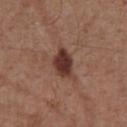Q: Was a biopsy performed?
A: imaged on a skin check; not biopsied
Q: What is the lesion's diameter?
A: about 4.5 mm
Q: Automated lesion metrics?
A: a lesion area of about 8 mm² and a shape eccentricity near 0.75; a border-irregularity index near 3.5/10, internal color variation of about 3 on a 0–10 scale, and a peripheral color-asymmetry measure near 1; a classifier nevus-likeness of about 90/100 and a lesion-detection confidence of about 100/100
Q: How was this image acquired?
A: 15 mm crop, total-body photography
Q: What is the anatomic site?
A: the chest
Q: How was the tile lit?
A: white-light
Q: What are the patient's age and sex?
A: male, about 55 years old